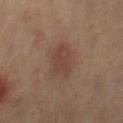notes — total-body-photography surveillance lesion; no biopsy | imaging modality — ~15 mm crop, total-body skin-cancer survey | size — ≈3.5 mm | TBP lesion metrics — lesion-presence confidence of about 100/100 | body site — the right thigh | subject — female, aged around 40.